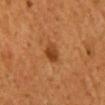Clinical impression:
The lesion was tiled from a total-body skin photograph and was not biopsied.
Acquisition and patient details:
Captured under cross-polarized illumination. The lesion is on the mid back. Longest diameter approximately 3 mm. The patient is a male approximately 45 years of age. This image is a 15 mm lesion crop taken from a total-body photograph.